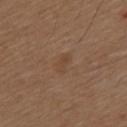Findings:
- notes — imaged on a skin check; not biopsied
- anatomic site — the mid back
- tile lighting — white-light illumination
- patient — male, aged around 75
- image source — 15 mm crop, total-body photography
- lesion size — about 2.5 mm
- automated metrics — a symmetry-axis asymmetry near 0.25; a border-irregularity rating of about 2.5/10, internal color variation of about 0 on a 0–10 scale, and a peripheral color-asymmetry measure near 0